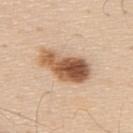tile lighting: white-light illumination
acquisition: ~15 mm tile from a whole-body skin photo
body site: the back
size: ~6 mm (longest diameter)
patient: male, approximately 60 years of age